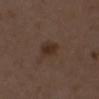<tbp_lesion>
<biopsy_status>not biopsied; imaged during a skin examination</biopsy_status>
<site>chest</site>
<automated_metrics>
  <area_mm2_approx>5.5</area_mm2_approx>
  <eccentricity>0.6</eccentricity>
  <shape_asymmetry>0.2</shape_asymmetry>
  <border_irregularity_0_10>2.0</border_irregularity_0_10>
  <peripheral_color_asymmetry>1.0</peripheral_color_asymmetry>
  <nevus_likeness_0_100>85</nevus_likeness_0_100>
</automated_metrics>
<patient>
  <sex>female</sex>
  <age_approx>50</age_approx>
</patient>
<lighting>white-light</lighting>
<lesion_size>
  <long_diameter_mm_approx>3.0</long_diameter_mm_approx>
</lesion_size>
<image>
  <source>total-body photography crop</source>
  <field_of_view_mm>15</field_of_view_mm>
</image>
</tbp_lesion>